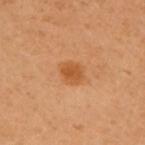Notes:
* notes — total-body-photography surveillance lesion; no biopsy
* imaging modality — total-body-photography crop, ~15 mm field of view
* body site — the chest
* tile lighting — cross-polarized illumination
* image-analysis metrics — a lesion color around L≈47 a*≈23 b*≈38 in CIELAB, roughly 8 lightness units darker than nearby skin, and a normalized border contrast of about 7; a border-irregularity rating of about 2/10, a color-variation rating of about 1.5/10, and radial color variation of about 0.5
* size — ~2.5 mm (longest diameter)
* patient — female, aged approximately 60